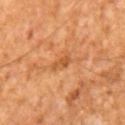<tbp_lesion>
  <biopsy_status>not biopsied; imaged during a skin examination</biopsy_status>
  <site>mid back</site>
  <automated_metrics>
    <eccentricity>0.9</eccentricity>
    <nevus_likeness_0_100>0</nevus_likeness_0_100>
    <lesion_detection_confidence_0_100>100</lesion_detection_confidence_0_100>
  </automated_metrics>
  <patient>
    <sex>male</sex>
    <age_approx>65</age_approx>
  </patient>
  <image>
    <source>total-body photography crop</source>
    <field_of_view_mm>15</field_of_view_mm>
  </image>
  <lighting>cross-polarized</lighting>
</tbp_lesion>